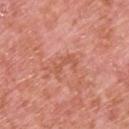notes: total-body-photography surveillance lesion; no biopsy | illumination: white-light | body site: the upper back | subject: male, aged 68–72 | image-analysis metrics: a footprint of about 5 mm², an eccentricity of roughly 0.9, and a symmetry-axis asymmetry near 0.55; a mean CIELAB color near L≈57 a*≈28 b*≈32 and roughly 7 lightness units darker than nearby skin; border irregularity of about 8.5 on a 0–10 scale | image: 15 mm crop, total-body photography.